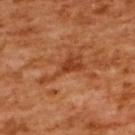Recorded during total-body skin imaging; not selected for excision or biopsy. A female subject about 55 years old. A lesion tile, about 15 mm wide, cut from a 3D total-body photograph. Imaged with cross-polarized lighting. Located on the upper back.